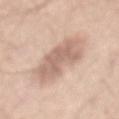This lesion was catalogued during total-body skin photography and was not selected for biopsy.
The total-body-photography lesion software estimated an automated nevus-likeness rating near 5 out of 100 and a detector confidence of about 100 out of 100 that the crop contains a lesion.
From the mid back.
The subject is a male roughly 45 years of age.
Cropped from a total-body skin-imaging series; the visible field is about 15 mm.
Captured under white-light illumination.